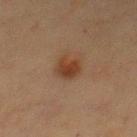Q: Was a biopsy performed?
A: imaged on a skin check; not biopsied
Q: Lesion location?
A: the front of the torso
Q: What lighting was used for the tile?
A: cross-polarized
Q: Automated lesion metrics?
A: an average lesion color of about L≈32 a*≈17 b*≈27 (CIELAB) and roughly 8 lightness units darker than nearby skin; a border-irregularity index near 3/10, a color-variation rating of about 2.5/10, and a peripheral color-asymmetry measure near 1; an automated nevus-likeness rating near 95 out of 100
Q: What is the imaging modality?
A: 15 mm crop, total-body photography
Q: Patient demographics?
A: female, aged 53 to 57